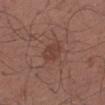Case summary:
- notes · no biopsy performed (imaged during a skin exam)
- imaging modality · ~15 mm crop, total-body skin-cancer survey
- body site · the right upper arm
- patient · male, in their mid- to late 30s
- diameter · about 3 mm
- illumination · white-light illumination